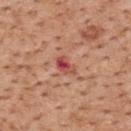No biopsy was performed on this lesion — it was imaged during a full skin examination and was not determined to be concerning.
A 15 mm close-up tile from a total-body photography series done for melanoma screening.
The lesion's longest dimension is about 2.5 mm.
A male subject aged 58–62.
The lesion is on the back.